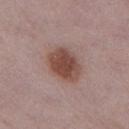No biopsy was performed on this lesion — it was imaged during a full skin examination and was not determined to be concerning. The lesion is on the left thigh. A female subject, aged around 30. Cropped from a whole-body photographic skin survey; the tile spans about 15 mm. The recorded lesion diameter is about 4.5 mm. The tile uses white-light illumination.